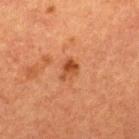Impression: The lesion was photographed on a routine skin check and not biopsied; there is no pathology result. Image and clinical context: A male patient, roughly 50 years of age. A 15 mm close-up extracted from a 3D total-body photography capture. The lesion is located on the upper back.